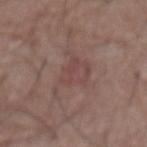notes = total-body-photography surveillance lesion; no biopsy
illumination = white-light illumination
lesion size = ≈6 mm
subject = male, in their mid- to late 50s
acquisition = ~15 mm crop, total-body skin-cancer survey
location = the abdomen